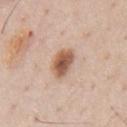Q: Was this lesion biopsied?
A: catalogued during a skin exam; not biopsied
Q: Patient demographics?
A: male, about 60 years old
Q: What is the anatomic site?
A: the chest
Q: Automated lesion metrics?
A: a lesion area of about 7.5 mm², an outline eccentricity of about 0.7 (0 = round, 1 = elongated), and a shape-asymmetry score of about 0.2 (0 = symmetric); a lesion–skin lightness drop of about 15 and a normalized lesion–skin contrast near 10
Q: What is the lesion's diameter?
A: ~3.5 mm (longest diameter)
Q: What is the imaging modality?
A: ~15 mm crop, total-body skin-cancer survey